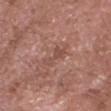Captured during whole-body skin photography for melanoma surveillance; the lesion was not biopsied.
A male patient in their mid- to late 70s.
The lesion is located on the head or neck.
Captured under white-light illumination.
The recorded lesion diameter is about 4 mm.
A region of skin cropped from a whole-body photographic capture, roughly 15 mm wide.